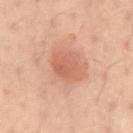<tbp_lesion>
  <biopsy_status>not biopsied; imaged during a skin examination</biopsy_status>
  <patient>
    <sex>male</sex>
    <age_approx>40</age_approx>
  </patient>
  <site>abdomen</site>
  <automated_metrics>
    <area_mm2_approx>10.0</area_mm2_approx>
    <shape_asymmetry>0.15</shape_asymmetry>
    <cielab_L>48</cielab_L>
    <cielab_a>21</cielab_a>
    <cielab_b>26</cielab_b>
    <vs_skin_darker_L>7.0</vs_skin_darker_L>
    <vs_skin_contrast_norm>5.5</vs_skin_contrast_norm>
    <border_irregularity_0_10>1.5</border_irregularity_0_10>
    <peripheral_color_asymmetry>1.0</peripheral_color_asymmetry>
  </automated_metrics>
  <image>
    <source>total-body photography crop</source>
    <field_of_view_mm>15</field_of_view_mm>
  </image>
  <lighting>cross-polarized</lighting>
</tbp_lesion>